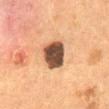| feature | finding |
|---|---|
| patient | female, roughly 50 years of age |
| image | 15 mm crop, total-body photography |
| body site | the abdomen |